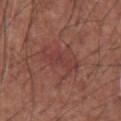| key | value |
|---|---|
| biopsy status | imaged on a skin check; not biopsied |
| subject | male, aged 73–77 |
| site | the chest |
| imaging modality | total-body-photography crop, ~15 mm field of view |
| lesion size | about 5.5 mm |